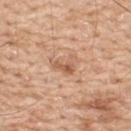Case summary:
– workup · catalogued during a skin exam; not biopsied
– location · the upper back
– patient · male, roughly 60 years of age
– diameter · ≈3 mm
– image · total-body-photography crop, ~15 mm field of view
– lighting · white-light